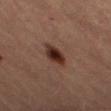imaging modality=~15 mm crop, total-body skin-cancer survey
subject=female, aged around 60
anatomic site=the left thigh